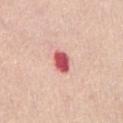Assessment:
The lesion was tiled from a total-body skin photograph and was not biopsied.
Clinical summary:
Cropped from a total-body skin-imaging series; the visible field is about 15 mm. A female subject aged 58 to 62. On the abdomen.